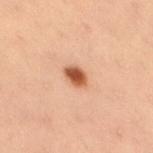Assessment: Captured during whole-body skin photography for melanoma surveillance; the lesion was not biopsied. Image and clinical context: A female patient, approximately 40 years of age. Imaged with cross-polarized lighting. The total-body-photography lesion software estimated a mean CIELAB color near L≈45 a*≈22 b*≈31, roughly 14 lightness units darker than nearby skin, and a lesion-to-skin contrast of about 11 (normalized; higher = more distinct). And it measured a nevus-likeness score of about 100/100 and lesion-presence confidence of about 100/100. Measured at roughly 2.5 mm in maximum diameter. A 15 mm close-up extracted from a 3D total-body photography capture. The lesion is on the right thigh.